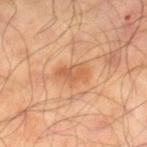notes = catalogued during a skin exam; not biopsied | site = the right lower leg | image source = total-body-photography crop, ~15 mm field of view | automated metrics = a border-irregularity index near 3.5/10 and internal color variation of about 3.5 on a 0–10 scale; an automated nevus-likeness rating near 50 out of 100 | subject = male, aged 63 to 67 | lighting = cross-polarized illumination.